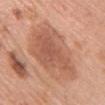Impression:
This lesion was catalogued during total-body skin photography and was not selected for biopsy.
Image and clinical context:
The patient is a female aged approximately 60. A lesion tile, about 15 mm wide, cut from a 3D total-body photograph. The lesion is on the chest.